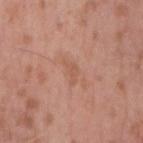follow-up — no biopsy performed (imaged during a skin exam) | patient — male, aged 53–57 | imaging modality — ~15 mm tile from a whole-body skin photo | tile lighting — white-light illumination | location — the right upper arm.